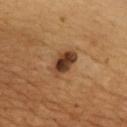Captured during whole-body skin photography for melanoma surveillance; the lesion was not biopsied. A male patient, approximately 45 years of age. The lesion is located on the chest. A 15 mm crop from a total-body photograph taken for skin-cancer surveillance.